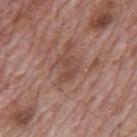The patient is a male aged 68–72.
Approximately 3 mm at its widest.
A 15 mm close-up extracted from a 3D total-body photography capture.
Located on the mid back.